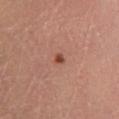follow-up: no biopsy performed (imaged during a skin exam); imaging modality: 15 mm crop, total-body photography; subject: female, in their mid- to late 30s; size: ≈1.5 mm; anatomic site: the left lower leg.